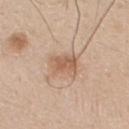Impression:
The lesion was tiled from a total-body skin photograph and was not biopsied.
Context:
Captured under white-light illumination. Cropped from a whole-body photographic skin survey; the tile spans about 15 mm. Located on the arm. Longest diameter approximately 3.5 mm. Automated image analysis of the tile measured a lesion area of about 6.5 mm², a shape eccentricity near 0.65, and two-axis asymmetry of about 0.2. And it measured roughly 10 lightness units darker than nearby skin and a normalized lesion–skin contrast near 6.5. The analysis additionally found a border-irregularity index near 2.5/10 and a within-lesion color-variation index near 3/10. It also reported a nevus-likeness score of about 75/100 and a detector confidence of about 100 out of 100 that the crop contains a lesion. A male subject, in their 30s.